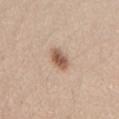{"biopsy_status": "not biopsied; imaged during a skin examination", "patient": {"sex": "female", "age_approx": 30}, "lighting": "white-light", "site": "lower back", "lesion_size": {"long_diameter_mm_approx": 2.5}, "image": {"source": "total-body photography crop", "field_of_view_mm": 15}, "automated_metrics": {"cielab_L": 56, "cielab_a": 18, "cielab_b": 29, "vs_skin_contrast_norm": 9.0, "border_irregularity_0_10": 2.0, "color_variation_0_10": 4.5, "peripheral_color_asymmetry": 1.5, "nevus_likeness_0_100": 95}}